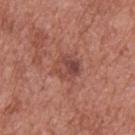Imaged during a routine full-body skin examination; the lesion was not biopsied and no histopathology is available.
A region of skin cropped from a whole-body photographic capture, roughly 15 mm wide.
The lesion is located on the upper back.
A male patient, about 70 years old.
About 3.5 mm across.
The tile uses white-light illumination.
The total-body-photography lesion software estimated a lesion color around L≈46 a*≈25 b*≈26 in CIELAB and a lesion-to-skin contrast of about 6.5 (normalized; higher = more distinct). It also reported border irregularity of about 2 on a 0–10 scale and peripheral color asymmetry of about 2.5.